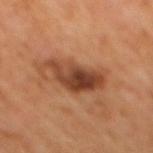  biopsy_status: not biopsied; imaged during a skin examination
  patient:
    sex: male
    age_approx: 65
  lesion_size:
    long_diameter_mm_approx: 5.5
  image:
    source: total-body photography crop
    field_of_view_mm: 15
  site: back
  lighting: cross-polarized